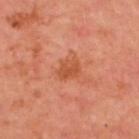notes = catalogued during a skin exam; not biopsied | location = the upper back | imaging modality = total-body-photography crop, ~15 mm field of view | patient = male, aged approximately 50.